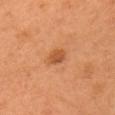This lesion was catalogued during total-body skin photography and was not selected for biopsy. The patient is a female aged 63 to 67. Captured under cross-polarized illumination. This image is a 15 mm lesion crop taken from a total-body photograph. The lesion is on the head or neck. Longest diameter approximately 2.5 mm.